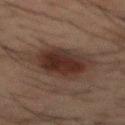Clinical impression: Recorded during total-body skin imaging; not selected for excision or biopsy. Clinical summary: A region of skin cropped from a whole-body photographic capture, roughly 15 mm wide. Located on the mid back. The patient is a male in their 50s. The lesion's longest dimension is about 5.5 mm. Imaged with cross-polarized lighting.